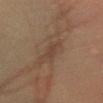Assessment:
This lesion was catalogued during total-body skin photography and was not selected for biopsy.
Context:
Located on the left forearm. This is a cross-polarized tile. A male subject aged 58 to 62. A lesion tile, about 15 mm wide, cut from a 3D total-body photograph. The lesion-visualizer software estimated a footprint of about 3 mm², an outline eccentricity of about 0.95 (0 = round, 1 = elongated), and a shape-asymmetry score of about 0.25 (0 = symmetric).